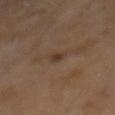notes — total-body-photography surveillance lesion; no biopsy
image source — ~15 mm crop, total-body skin-cancer survey
subject — male, roughly 70 years of age
anatomic site — the mid back
TBP lesion metrics — an area of roughly 1.5 mm², a shape eccentricity near 0.6, and a shape-asymmetry score of about 0.3 (0 = symmetric); a lesion–skin lightness drop of about 8 and a lesion-to-skin contrast of about 7.5 (normalized; higher = more distinct)
tile lighting — cross-polarized
lesion size — ~1.5 mm (longest diameter)